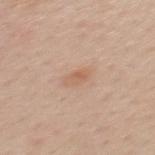Longest diameter approximately 3 mm. Automated image analysis of the tile measured a lesion area of about 4 mm² and a shape-asymmetry score of about 0.3 (0 = symmetric). The analysis additionally found lesion-presence confidence of about 100/100. A 15 mm close-up tile from a total-body photography series done for melanoma screening. The lesion is located on the upper back. Captured under white-light illumination. A female subject, roughly 40 years of age.